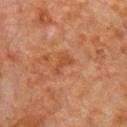{"biopsy_status": "not biopsied; imaged during a skin examination", "automated_metrics": {"nevus_likeness_0_100": 0, "lesion_detection_confidence_0_100": 100}, "site": "chest", "patient": {"sex": "male", "age_approx": 80}, "lighting": "cross-polarized", "image": {"source": "total-body photography crop", "field_of_view_mm": 15}}